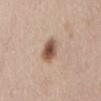* follow-up — imaged on a skin check; not biopsied
* image-analysis metrics — about 15 CIELAB-L* units darker than the surrounding skin; an automated nevus-likeness rating near 100 out of 100
* imaging modality — 15 mm crop, total-body photography
* site — the abdomen
* subject — male, aged approximately 50